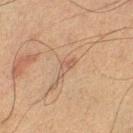<record>
  <biopsy_status>not biopsied; imaged during a skin examination</biopsy_status>
  <site>left lower leg</site>
  <patient>
    <sex>male</sex>
    <age_approx>60</age_approx>
  </patient>
  <lighting>cross-polarized</lighting>
  <lesion_size>
    <long_diameter_mm_approx>3.0</long_diameter_mm_approx>
  </lesion_size>
  <image>
    <source>total-body photography crop</source>
    <field_of_view_mm>15</field_of_view_mm>
  </image>
</record>